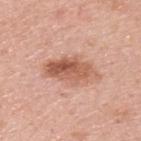| key | value |
|---|---|
| workup | catalogued during a skin exam; not biopsied |
| patient | male, in their mid- to late 60s |
| diameter | ~6 mm (longest diameter) |
| image source | ~15 mm crop, total-body skin-cancer survey |
| body site | the back |
| TBP lesion metrics | an average lesion color of about L≈58 a*≈24 b*≈31 (CIELAB), roughly 13 lightness units darker than nearby skin, and a normalized lesion–skin contrast near 8.5; border irregularity of about 3.5 on a 0–10 scale, a color-variation rating of about 7.5/10, and radial color variation of about 3 |
| illumination | white-light illumination |